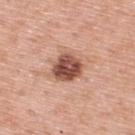Q: Was this lesion biopsied?
A: catalogued during a skin exam; not biopsied
Q: What is the imaging modality?
A: ~15 mm crop, total-body skin-cancer survey
Q: What is the lesion's diameter?
A: about 4 mm
Q: What lighting was used for the tile?
A: white-light
Q: Where on the body is the lesion?
A: the back
Q: Patient demographics?
A: male, about 60 years old